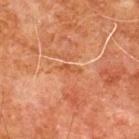Clinical impression:
No biopsy was performed on this lesion — it was imaged during a full skin examination and was not determined to be concerning.
Context:
The subject is a male roughly 80 years of age. The tile uses cross-polarized illumination. The recorded lesion diameter is about 2.5 mm. A lesion tile, about 15 mm wide, cut from a 3D total-body photograph. The lesion is located on the upper back.